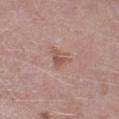Assessment:
Part of a total-body skin-imaging series; this lesion was reviewed on a skin check and was not flagged for biopsy.
Background:
The lesion's longest dimension is about 3 mm. A male subject, roughly 50 years of age. From the leg. This image is a 15 mm lesion crop taken from a total-body photograph.